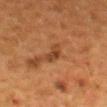Clinical impression:
Captured during whole-body skin photography for melanoma surveillance; the lesion was not biopsied.
Context:
A lesion tile, about 15 mm wide, cut from a 3D total-body photograph. This is a cross-polarized tile. On the back. Approximately 3 mm at its widest. The lesion-visualizer software estimated a lesion color around L≈35 a*≈20 b*≈31 in CIELAB and about 8 CIELAB-L* units darker than the surrounding skin. The software also gave a border-irregularity rating of about 3.5/10, internal color variation of about 3.5 on a 0–10 scale, and radial color variation of about 1. A female patient, aged 48 to 52.